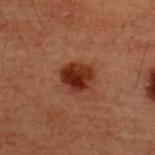biopsy status: imaged on a skin check; not biopsied | subject: male, about 60 years old | anatomic site: the back | image: 15 mm crop, total-body photography.